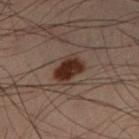| key | value |
|---|---|
| workup | catalogued during a skin exam; not biopsied |
| image source | 15 mm crop, total-body photography |
| patient | male, in their mid-50s |
| anatomic site | the left lower leg |